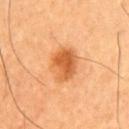Captured during whole-body skin photography for melanoma surveillance; the lesion was not biopsied. A lesion tile, about 15 mm wide, cut from a 3D total-body photograph. Automated image analysis of the tile measured a lesion area of about 10 mm², a shape eccentricity near 0.65, and a shape-asymmetry score of about 0.15 (0 = symmetric). It also reported a border-irregularity rating of about 2/10, a color-variation rating of about 5/10, and peripheral color asymmetry of about 1.5. The software also gave an automated nevus-likeness rating near 100 out of 100 and a detector confidence of about 100 out of 100 that the crop contains a lesion. Captured under cross-polarized illumination. From the chest. A male subject, roughly 60 years of age. Longest diameter approximately 4 mm.